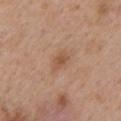Recorded during total-body skin imaging; not selected for excision or biopsy. A female subject aged 38–42. The lesion is located on the left upper arm. A close-up tile cropped from a whole-body skin photograph, about 15 mm across.